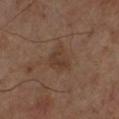biopsy_status: not biopsied; imaged during a skin examination
image:
  source: total-body photography crop
  field_of_view_mm: 15
site: left lower leg
lighting: cross-polarized
lesion_size:
  long_diameter_mm_approx: 3.5
patient:
  sex: male
  age_approx: 65